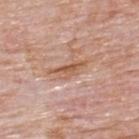Part of a total-body skin-imaging series; this lesion was reviewed on a skin check and was not flagged for biopsy. The subject is a male aged 73–77. A 15 mm crop from a total-body photograph taken for skin-cancer surveillance. Located on the back. Imaged with white-light lighting.